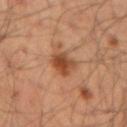image:
  source: total-body photography crop
  field_of_view_mm: 15
site: left forearm
patient:
  sex: male
  age_approx: 35